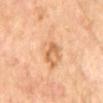follow-up: total-body-photography surveillance lesion; no biopsy
lighting: cross-polarized illumination
image source: ~15 mm crop, total-body skin-cancer survey
anatomic site: the back
subject: male, roughly 70 years of age
TBP lesion metrics: an eccentricity of roughly 0.9 and a shape-asymmetry score of about 0.25 (0 = symmetric); a nevus-likeness score of about 0/100